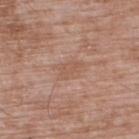Imaged during a routine full-body skin examination; the lesion was not biopsied and no histopathology is available. A region of skin cropped from a whole-body photographic capture, roughly 15 mm wide. From the upper back. Imaged with white-light lighting. A male patient aged 48–52. About 3 mm across.